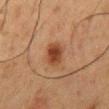Imaged during a routine full-body skin examination; the lesion was not biopsied and no histopathology is available. Located on the mid back. The total-body-photography lesion software estimated a lesion area of about 6.5 mm² and a shape eccentricity near 0.7. It also reported a border-irregularity rating of about 2/10, internal color variation of about 3 on a 0–10 scale, and a peripheral color-asymmetry measure near 1. The software also gave lesion-presence confidence of about 100/100. A 15 mm crop from a total-body photograph taken for skin-cancer surveillance. Measured at roughly 3 mm in maximum diameter. The tile uses cross-polarized illumination. The subject is a male aged 58 to 62.